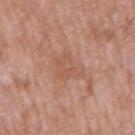Findings:
– notes: imaged on a skin check; not biopsied
– lesion diameter: ≈4 mm
– subject: male, roughly 60 years of age
– image source: ~15 mm tile from a whole-body skin photo
– body site: the right upper arm
– TBP lesion metrics: a footprint of about 7.5 mm², an outline eccentricity of about 0.75 (0 = round, 1 = elongated), and a shape-asymmetry score of about 0.45 (0 = symmetric); a border-irregularity rating of about 5/10; a nevus-likeness score of about 0/100 and a detector confidence of about 100 out of 100 that the crop contains a lesion
– lighting: white-light illumination